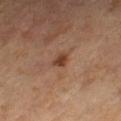* workup · total-body-photography surveillance lesion; no biopsy
* acquisition · ~15 mm tile from a whole-body skin photo
* lesion size · ≈2 mm
* tile lighting · cross-polarized
* patient · female, in their 70s
* body site · the right lower leg